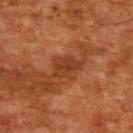Impression:
No biopsy was performed on this lesion — it was imaged during a full skin examination and was not determined to be concerning.
Clinical summary:
The lesion-visualizer software estimated a footprint of about 6 mm² and an eccentricity of roughly 0.65. It also reported border irregularity of about 3.5 on a 0–10 scale, a within-lesion color-variation index near 2.5/10, and a peripheral color-asymmetry measure near 1. The software also gave a nevus-likeness score of about 0/100 and a lesion-detection confidence of about 100/100. A 15 mm crop from a total-body photograph taken for skin-cancer surveillance. The patient is a male roughly 65 years of age.